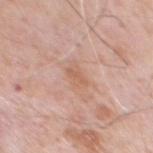Case summary:
• workup: catalogued during a skin exam; not biopsied
• subject: male, aged approximately 80
• anatomic site: the chest
• illumination: white-light
• image source: ~15 mm tile from a whole-body skin photo
• TBP lesion metrics: a footprint of about 4 mm², a shape eccentricity near 0.85, and a shape-asymmetry score of about 0.2 (0 = symmetric); a border-irregularity rating of about 2.5/10, a within-lesion color-variation index near 2/10, and radial color variation of about 0.5